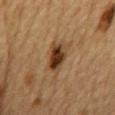Imaged during a routine full-body skin examination; the lesion was not biopsied and no histopathology is available.
A male subject, in their mid- to late 80s or older.
The lesion is located on the mid back.
The tile uses cross-polarized illumination.
Longest diameter approximately 4 mm.
Cropped from a whole-body photographic skin survey; the tile spans about 15 mm.
An algorithmic analysis of the crop reported a lesion area of about 7.5 mm², an outline eccentricity of about 0.8 (0 = round, 1 = elongated), and two-axis asymmetry of about 0.3. It also reported a classifier nevus-likeness of about 100/100 and lesion-presence confidence of about 100/100.